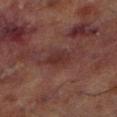Part of a total-body skin-imaging series; this lesion was reviewed on a skin check and was not flagged for biopsy. The lesion is located on the left lower leg. The total-body-photography lesion software estimated an average lesion color of about L≈24 a*≈18 b*≈17 (CIELAB) and a normalized border contrast of about 6.5. The analysis additionally found lesion-presence confidence of about 100/100. Imaged with cross-polarized lighting. The subject is a male aged 68–72. This image is a 15 mm lesion crop taken from a total-body photograph. Approximately 3 mm at its widest.